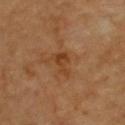workup: catalogued during a skin exam; not biopsied | size: ~3 mm (longest diameter) | image: ~15 mm tile from a whole-body skin photo | patient: roughly 60 years of age | location: the upper back | tile lighting: cross-polarized.